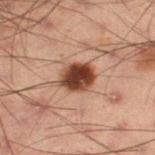{"biopsy_status": "not biopsied; imaged during a skin examination", "site": "left thigh", "image": {"source": "total-body photography crop", "field_of_view_mm": 15}, "lighting": "cross-polarized", "patient": {"sex": "male", "age_approx": 55}}